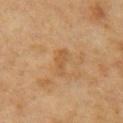follow-up=catalogued during a skin exam; not biopsied
subject=female, approximately 60 years of age
body site=the right upper arm
illumination=cross-polarized illumination
image source=~15 mm tile from a whole-body skin photo